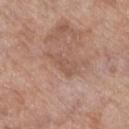Case summary:
* notes: catalogued during a skin exam; not biopsied
* lighting: white-light
* size: ≈4 mm
* subject: female, aged 83 or older
* image source: ~15 mm tile from a whole-body skin photo
* site: the leg